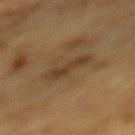patient:
  sex: male
  age_approx: 85+
image:
  source: total-body photography crop
  field_of_view_mm: 15
site: mid back